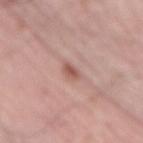Q: Was a biopsy performed?
A: imaged on a skin check; not biopsied
Q: What did automated image analysis measure?
A: an eccentricity of roughly 0.9 and a shape-asymmetry score of about 0.25 (0 = symmetric); a border-irregularity rating of about 3/10 and radial color variation of about 0; a detector confidence of about 100 out of 100 that the crop contains a lesion
Q: What is the anatomic site?
A: the mid back
Q: What is the imaging modality?
A: 15 mm crop, total-body photography
Q: How large is the lesion?
A: ≈3 mm
Q: Who is the patient?
A: male, approximately 65 years of age
Q: Illumination type?
A: white-light